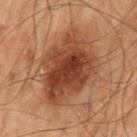Recorded during total-body skin imaging; not selected for excision or biopsy. A 15 mm crop from a total-body photograph taken for skin-cancer surveillance. About 7 mm across. The lesion is on the mid back. A male subject, aged around 70. Captured under cross-polarized illumination.